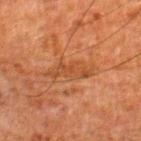This lesion was catalogued during total-body skin photography and was not selected for biopsy. A male subject, in their 80s. A close-up tile cropped from a whole-body skin photograph, about 15 mm across. Automated image analysis of the tile measured a lesion area of about 8 mm², an eccentricity of roughly 0.9, and a shape-asymmetry score of about 0.35 (0 = symmetric). The software also gave a border-irregularity index near 5/10, a color-variation rating of about 2.5/10, and radial color variation of about 0.5. About 5 mm across. On the right lower leg. Imaged with cross-polarized lighting.